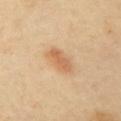No biopsy was performed on this lesion — it was imaged during a full skin examination and was not determined to be concerning.
Located on the left upper arm.
A female patient, approximately 40 years of age.
A lesion tile, about 15 mm wide, cut from a 3D total-body photograph.
Captured under cross-polarized illumination.
Automated tile analysis of the lesion measured a footprint of about 6.5 mm². It also reported a lesion–skin lightness drop of about 10 and a normalized border contrast of about 6.5.